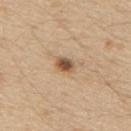notes = no biopsy performed (imaged during a skin exam)
lighting = white-light illumination
site = the mid back
acquisition = 15 mm crop, total-body photography
size = about 2.5 mm
patient = male, in their 70s
image-analysis metrics = an eccentricity of roughly 0.75 and two-axis asymmetry of about 0.2; a border-irregularity rating of about 2/10 and internal color variation of about 4.5 on a 0–10 scale; a lesion-detection confidence of about 100/100